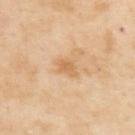{
  "biopsy_status": "not biopsied; imaged during a skin examination",
  "site": "upper back",
  "lighting": "cross-polarized",
  "automated_metrics": {
    "area_mm2_approx": 3.5,
    "eccentricity": 0.8,
    "shape_asymmetry": 0.35,
    "color_variation_0_10": 1.5,
    "peripheral_color_asymmetry": 0.5,
    "nevus_likeness_0_100": 0,
    "lesion_detection_confidence_0_100": 100
  },
  "patient": {
    "sex": "male",
    "age_approx": 55
  },
  "image": {
    "source": "total-body photography crop",
    "field_of_view_mm": 15
  },
  "lesion_size": {
    "long_diameter_mm_approx": 3.0
  }
}